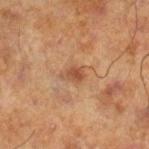Notes:
– notes · imaged on a skin check; not biopsied
– automated lesion analysis · an eccentricity of roughly 0.75 and two-axis asymmetry of about 0.25; an average lesion color of about L≈42 a*≈19 b*≈29 (CIELAB), roughly 8 lightness units darker than nearby skin, and a normalized lesion–skin contrast near 6.5; a border-irregularity rating of about 2.5/10, a color-variation rating of about 3/10, and peripheral color asymmetry of about 1
– patient · male, about 70 years old
– lighting · cross-polarized
– site · the right lower leg
– imaging modality · ~15 mm crop, total-body skin-cancer survey